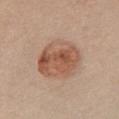<case>
  <biopsy_status>not biopsied; imaged during a skin examination</biopsy_status>
  <site>front of the torso</site>
  <image>
    <source>total-body photography crop</source>
    <field_of_view_mm>15</field_of_view_mm>
  </image>
  <lesion_size>
    <long_diameter_mm_approx>5.5</long_diameter_mm_approx>
  </lesion_size>
  <patient>
    <sex>female</sex>
    <age_approx>35</age_approx>
  </patient>
  <lighting>white-light</lighting>
  <automated_metrics>
    <area_mm2_approx>21.0</area_mm2_approx>
    <eccentricity>0.45</eccentricity>
    <shape_asymmetry>0.1</shape_asymmetry>
    <cielab_L>55</cielab_L>
    <cielab_a>21</cielab_a>
    <cielab_b>30</cielab_b>
    <vs_skin_contrast_norm>7.5</vs_skin_contrast_norm>
    <nevus_likeness_0_100>90</nevus_likeness_0_100>
    <lesion_detection_confidence_0_100>100</lesion_detection_confidence_0_100>
  </automated_metrics>
</case>